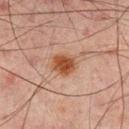follow-up = imaged on a skin check; not biopsied
location = the mid back
subject = male, aged 63 to 67
lesion size = about 3 mm
acquisition = 15 mm crop, total-body photography